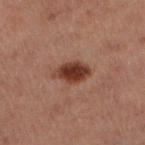Impression: No biopsy was performed on this lesion — it was imaged during a full skin examination and was not determined to be concerning. Clinical summary: About 4 mm across. The lesion is on the left lower leg. This is a cross-polarized tile. The lesion-visualizer software estimated a mean CIELAB color near L≈28 a*≈18 b*≈21, a lesion–skin lightness drop of about 11, and a lesion-to-skin contrast of about 11 (normalized; higher = more distinct). The software also gave a border-irregularity rating of about 2/10 and radial color variation of about 1. It also reported a nevus-likeness score of about 100/100 and a detector confidence of about 100 out of 100 that the crop contains a lesion. The patient is a male roughly 60 years of age. Cropped from a total-body skin-imaging series; the visible field is about 15 mm.